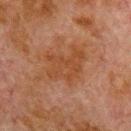Notes:
– follow-up — catalogued during a skin exam; not biopsied
– subject — male, aged 78–82
– size — about 4.5 mm
– image — ~15 mm tile from a whole-body skin photo
– automated metrics — border irregularity of about 5 on a 0–10 scale, a within-lesion color-variation index near 2.5/10, and a peripheral color-asymmetry measure near 1; an automated nevus-likeness rating near 0 out of 100 and lesion-presence confidence of about 100/100
– site — the chest
– lighting — cross-polarized illumination